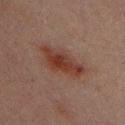Recorded during total-body skin imaging; not selected for excision or biopsy. A male patient aged around 30. The lesion's longest dimension is about 5.5 mm. Imaged with cross-polarized lighting. A region of skin cropped from a whole-body photographic capture, roughly 15 mm wide. From the chest.